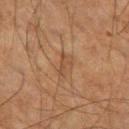From the right thigh.
A close-up tile cropped from a whole-body skin photograph, about 15 mm across.
The patient is a male about 60 years old.